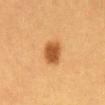  biopsy_status: not biopsied; imaged during a skin examination
  lighting: cross-polarized
  image:
    source: total-body photography crop
    field_of_view_mm: 15
  site: abdomen
  patient:
    sex: female
    age_approx: 40
  automated_metrics:
    vs_skin_darker_L: 12.0
    vs_skin_contrast_norm: 9.5